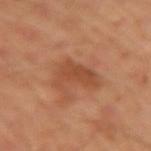| field | value |
|---|---|
| subject | male, roughly 45 years of age |
| imaging modality | ~15 mm tile from a whole-body skin photo |
| diameter | about 4 mm |
| site | the arm |